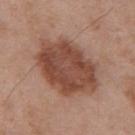Clinical impression: Part of a total-body skin-imaging series; this lesion was reviewed on a skin check and was not flagged for biopsy. Acquisition and patient details: The lesion's longest dimension is about 8.5 mm. Automated tile analysis of the lesion measured a footprint of about 34 mm², a shape eccentricity near 0.75, and two-axis asymmetry of about 0.2. It also reported a within-lesion color-variation index near 4.5/10 and peripheral color asymmetry of about 1.5. And it measured a nevus-likeness score of about 35/100 and lesion-presence confidence of about 100/100. The lesion is on the upper back. The subject is a male aged around 55. A lesion tile, about 15 mm wide, cut from a 3D total-body photograph.